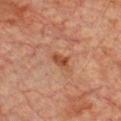Captured during whole-body skin photography for melanoma surveillance; the lesion was not biopsied.
From the chest.
A 15 mm crop from a total-body photograph taken for skin-cancer surveillance.
The lesion-visualizer software estimated a lesion area of about 4 mm², an outline eccentricity of about 0.85 (0 = round, 1 = elongated), and two-axis asymmetry of about 0.35. And it measured a mean CIELAB color near L≈40 a*≈22 b*≈29, a lesion–skin lightness drop of about 8, and a normalized border contrast of about 7.5.
A male patient roughly 75 years of age.
The lesion's longest dimension is about 3 mm.